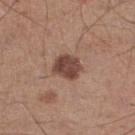notes: total-body-photography surveillance lesion; no biopsy
tile lighting: white-light
size: about 3.5 mm
image: ~15 mm crop, total-body skin-cancer survey
body site: the right lower leg
subject: male, aged approximately 60